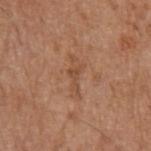No biopsy was performed on this lesion — it was imaged during a full skin examination and was not determined to be concerning.
Imaged with white-light lighting.
A 15 mm close-up extracted from a 3D total-body photography capture.
On the arm.
The lesion's longest dimension is about 4 mm.
The patient is a male approximately 65 years of age.